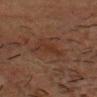Q: Is there a histopathology result?
A: catalogued during a skin exam; not biopsied